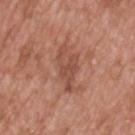{"biopsy_status": "not biopsied; imaged during a skin examination", "patient": {"sex": "male", "age_approx": 50}, "site": "upper back", "image": {"source": "total-body photography crop", "field_of_view_mm": 15}}